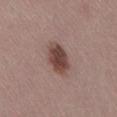A female subject aged around 45. A 15 mm crop from a total-body photograph taken for skin-cancer surveillance. About 4 mm across. The lesion is on the leg.